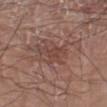| feature | finding |
|---|---|
| biopsy status | no biopsy performed (imaged during a skin exam) |
| image source | total-body-photography crop, ~15 mm field of view |
| patient | male, approximately 80 years of age |
| size | ~4.5 mm (longest diameter) |
| lighting | white-light illumination |
| location | the right arm |
| automated lesion analysis | a border-irregularity index near 5.5/10 and a within-lesion color-variation index near 1.5/10; an automated nevus-likeness rating near 0 out of 100 and a detector confidence of about 85 out of 100 that the crop contains a lesion |